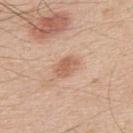Impression: Part of a total-body skin-imaging series; this lesion was reviewed on a skin check and was not flagged for biopsy. Context: The subject is a male approximately 50 years of age. An algorithmic analysis of the crop reported about 10 CIELAB-L* units darker than the surrounding skin and a lesion-to-skin contrast of about 6.5 (normalized; higher = more distinct). A region of skin cropped from a whole-body photographic capture, roughly 15 mm wide. From the upper back.